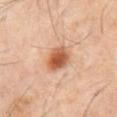biopsy status: no biopsy performed (imaged during a skin exam) | site: the abdomen | automated metrics: a footprint of about 8 mm², an outline eccentricity of about 0.75 (0 = round, 1 = elongated), and a shape-asymmetry score of about 0.2 (0 = symmetric); a border-irregularity index near 2/10 and peripheral color asymmetry of about 2 | tile lighting: cross-polarized | lesion size: ~4 mm (longest diameter) | subject: male, about 60 years old | image: ~15 mm crop, total-body skin-cancer survey.